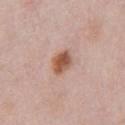Assessment: The lesion was tiled from a total-body skin photograph and was not biopsied. Image and clinical context: Longest diameter approximately 2.5 mm. A 15 mm crop from a total-body photograph taken for skin-cancer surveillance. From the front of the torso. Imaged with white-light lighting. An algorithmic analysis of the crop reported an area of roughly 5.5 mm² and an outline eccentricity of about 0.5 (0 = round, 1 = elongated). The software also gave a lesion color around L≈54 a*≈22 b*≈30 in CIELAB and a lesion-to-skin contrast of about 10 (normalized; higher = more distinct). And it measured border irregularity of about 1.5 on a 0–10 scale, internal color variation of about 4 on a 0–10 scale, and radial color variation of about 1.5. A male patient, approximately 40 years of age.